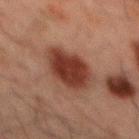Captured during whole-body skin photography for melanoma surveillance; the lesion was not biopsied.
On the back.
The tile uses cross-polarized illumination.
Automated tile analysis of the lesion measured a footprint of about 14 mm², an outline eccentricity of about 0.75 (0 = round, 1 = elongated), and two-axis asymmetry of about 0.2. It also reported a mean CIELAB color near L≈27 a*≈21 b*≈23 and a lesion–skin lightness drop of about 12. The analysis additionally found border irregularity of about 2 on a 0–10 scale and peripheral color asymmetry of about 0.5. And it measured a nevus-likeness score of about 100/100 and a lesion-detection confidence of about 100/100.
This image is a 15 mm lesion crop taken from a total-body photograph.
The subject is a male aged around 50.
Measured at roughly 5 mm in maximum diameter.